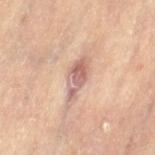workup=imaged on a skin check; not biopsied
subject=female, aged around 65
lighting=cross-polarized
imaging modality=~15 mm tile from a whole-body skin photo
body site=the left thigh
diameter=~5.5 mm (longest diameter)